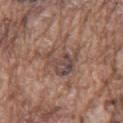Assessment: Part of a total-body skin-imaging series; this lesion was reviewed on a skin check and was not flagged for biopsy. Background: The subject is a male in their mid- to late 70s. A 15 mm crop from a total-body photograph taken for skin-cancer surveillance. The tile uses white-light illumination. The lesion is located on the chest.